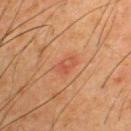Findings:
• imaging modality: ~15 mm crop, total-body skin-cancer survey
• patient: male, approximately 35 years of age
• body site: the upper back